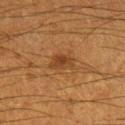workup: no biopsy performed (imaged during a skin exam)
body site: the right lower leg
image: total-body-photography crop, ~15 mm field of view
lesion size: ~3.5 mm (longest diameter)
illumination: cross-polarized illumination
TBP lesion metrics: a lesion area of about 5.5 mm², a shape eccentricity near 0.85, and a symmetry-axis asymmetry near 0.3; a lesion color around L≈35 a*≈19 b*≈32 in CIELAB and a lesion–skin lightness drop of about 8
patient: male, in their 60s